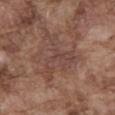Impression:
Captured during whole-body skin photography for melanoma surveillance; the lesion was not biopsied.
Clinical summary:
From the front of the torso. Captured under white-light illumination. A close-up tile cropped from a whole-body skin photograph, about 15 mm across. About 6.5 mm across. An algorithmic analysis of the crop reported an area of roughly 15 mm², an outline eccentricity of about 0.65 (0 = round, 1 = elongated), and a shape-asymmetry score of about 0.75 (0 = symmetric). And it measured a border-irregularity rating of about 10/10, a color-variation rating of about 3/10, and radial color variation of about 1. A male patient in their mid-70s.